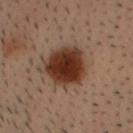{
  "biopsy_status": "not biopsied; imaged during a skin examination",
  "site": "head or neck",
  "patient": {
    "sex": "male",
    "age_approx": 30
  },
  "lighting": "cross-polarized",
  "image": {
    "source": "total-body photography crop",
    "field_of_view_mm": 15
  },
  "lesion_size": {
    "long_diameter_mm_approx": 5.0
  }
}